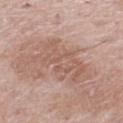  lesion_size:
    long_diameter_mm_approx: 6.0
  automated_metrics:
    area_mm2_approx: 17.0
    eccentricity: 0.8
    shape_asymmetry: 0.35
    cielab_L: 57
    cielab_a: 20
    cielab_b: 26
    vs_skin_darker_L: 7.0
    border_irregularity_0_10: 7.5
    color_variation_0_10: 3.0
    peripheral_color_asymmetry: 1.0
    nevus_likeness_0_100: 0
    lesion_detection_confidence_0_100: 100
  lighting: white-light
  image:
    source: total-body photography crop
    field_of_view_mm: 15
  site: leg
  patient:
    sex: female
    age_approx: 65